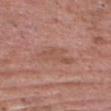Context: A male subject approximately 60 years of age. This is a white-light tile. About 4.5 mm across. This image is a 15 mm lesion crop taken from a total-body photograph. Located on the head or neck.